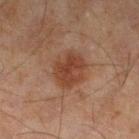follow-up: imaged on a skin check; not biopsied
lighting: cross-polarized illumination
acquisition: ~15 mm crop, total-body skin-cancer survey
automated metrics: a shape eccentricity near 0.6 and a symmetry-axis asymmetry near 0.15; about 8 CIELAB-L* units darker than the surrounding skin and a lesion-to-skin contrast of about 7.5 (normalized; higher = more distinct); an automated nevus-likeness rating near 50 out of 100 and a lesion-detection confidence of about 100/100
anatomic site: the left lower leg
subject: male, about 45 years old
lesion size: ~4.5 mm (longest diameter)